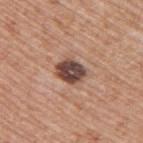Acquisition and patient details: A male patient aged 68–72. A 15 mm close-up extracted from a 3D total-body photography capture. This is a white-light tile. Automated tile analysis of the lesion measured a color-variation rating of about 4.5/10 and a peripheral color-asymmetry measure near 1.5. It also reported an automated nevus-likeness rating near 5 out of 100. The lesion is on the upper back.